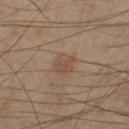Recorded during total-body skin imaging; not selected for excision or biopsy.
The tile uses cross-polarized illumination.
The lesion is located on the leg.
The lesion's longest dimension is about 3 mm.
A male subject aged around 55.
A close-up tile cropped from a whole-body skin photograph, about 15 mm across.
An algorithmic analysis of the crop reported a border-irregularity rating of about 2/10. The software also gave an automated nevus-likeness rating near 15 out of 100.